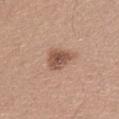follow-up — no biopsy performed (imaged during a skin exam) | subject — male, about 25 years old | imaging modality — ~15 mm crop, total-body skin-cancer survey | diameter — ≈3.5 mm | anatomic site — the chest | automated metrics — an area of roughly 7 mm² and a shape eccentricity near 0.7; border irregularity of about 3 on a 0–10 scale, internal color variation of about 3 on a 0–10 scale, and peripheral color asymmetry of about 1 | illumination — white-light illumination.